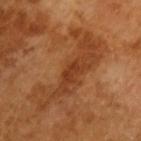The lesion was photographed on a routine skin check and not biopsied; there is no pathology result. Cropped from a total-body skin-imaging series; the visible field is about 15 mm. Captured under cross-polarized illumination. Automated image analysis of the tile measured a footprint of about 27 mm², an eccentricity of roughly 0.95, and a shape-asymmetry score of about 0.55 (0 = symmetric). The software also gave roughly 8 lightness units darker than nearby skin. It also reported a border-irregularity index near 9/10 and a within-lesion color-variation index near 3.5/10. The patient is a male aged 63 to 67. Measured at roughly 10.5 mm in maximum diameter.